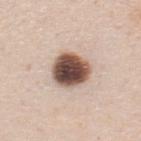| feature | finding |
|---|---|
| notes | no biopsy performed (imaged during a skin exam) |
| lesion size | about 4.5 mm |
| patient | male, aged 33 to 37 |
| body site | the upper back |
| acquisition | ~15 mm tile from a whole-body skin photo |
| illumination | white-light |
| automated lesion analysis | a mean CIELAB color near L≈51 a*≈17 b*≈24, about 24 CIELAB-L* units darker than the surrounding skin, and a normalized border contrast of about 15; a border-irregularity rating of about 1.5/10, a within-lesion color-variation index near 8.5/10, and a peripheral color-asymmetry measure near 2 |